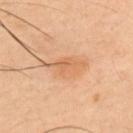About 5 mm across. An algorithmic analysis of the crop reported an eccentricity of roughly 0.9 and two-axis asymmetry of about 0.35. The analysis additionally found a lesion color around L≈63 a*≈22 b*≈38 in CIELAB, about 8 CIELAB-L* units darker than the surrounding skin, and a lesion-to-skin contrast of about 5.5 (normalized; higher = more distinct). The software also gave an automated nevus-likeness rating near 5 out of 100 and a lesion-detection confidence of about 100/100. From the left upper arm. Imaged with cross-polarized lighting. A male subject about 40 years old. Cropped from a whole-body photographic skin survey; the tile spans about 15 mm.